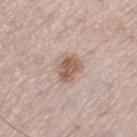patient=male, aged 53 to 57 | tile lighting=white-light | lesion size=≈3.5 mm | TBP lesion metrics=a mean CIELAB color near L≈58 a*≈17 b*≈26, roughly 11 lightness units darker than nearby skin, and a normalized border contrast of about 8 | acquisition=~15 mm crop, total-body skin-cancer survey | anatomic site=the left thigh.